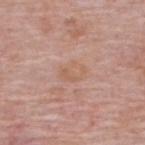Approximately 3 mm at its widest. Automated tile analysis of the lesion measured a mean CIELAB color near L≈60 a*≈20 b*≈30. The software also gave border irregularity of about 2.5 on a 0–10 scale and radial color variation of about 1. It also reported a classifier nevus-likeness of about 0/100 and a lesion-detection confidence of about 100/100. A male subject in their mid- to late 60s. Cropped from a total-body skin-imaging series; the visible field is about 15 mm. From the upper back.